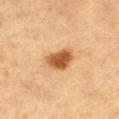Q: What is the anatomic site?
A: the left thigh
Q: What lighting was used for the tile?
A: cross-polarized illumination
Q: How large is the lesion?
A: about 4 mm
Q: What kind of image is this?
A: ~15 mm crop, total-body skin-cancer survey
Q: What are the patient's age and sex?
A: female, aged approximately 55